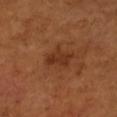Acquisition and patient details:
The lesion is on the right forearm. Automated image analysis of the tile measured a lesion color around L≈34 a*≈25 b*≈33 in CIELAB, a lesion–skin lightness drop of about 8, and a normalized lesion–skin contrast near 7. And it measured a color-variation rating of about 2.5/10 and radial color variation of about 0.5. About 3 mm across. The tile uses cross-polarized illumination. A 15 mm crop from a total-body photograph taken for skin-cancer surveillance. A female subject in their mid- to late 50s.